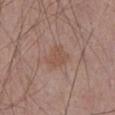Context:
The subject is a male aged 68 to 72. The tile uses white-light illumination. About 3 mm across. This image is a 15 mm lesion crop taken from a total-body photograph. From the abdomen. An algorithmic analysis of the crop reported an outline eccentricity of about 0.65 (0 = round, 1 = elongated). And it measured an average lesion color of about L≈51 a*≈19 b*≈27 (CIELAB) and about 6 CIELAB-L* units darker than the surrounding skin. It also reported a border-irregularity rating of about 3.5/10, a color-variation rating of about 1.5/10, and radial color variation of about 0.5. And it measured an automated nevus-likeness rating near 0 out of 100 and a detector confidence of about 100 out of 100 that the crop contains a lesion.